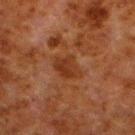A lesion tile, about 15 mm wide, cut from a 3D total-body photograph.
The lesion is located on the left lower leg.
Imaged with cross-polarized lighting.
The patient is a male aged 78–82.